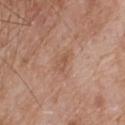Q: Was this lesion biopsied?
A: imaged on a skin check; not biopsied